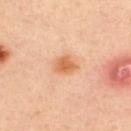Imaged during a routine full-body skin examination; the lesion was not biopsied and no histopathology is available.
A male subject aged 33–37.
Approximately 2.5 mm at its widest.
The lesion is on the upper back.
The total-body-photography lesion software estimated a lesion color around L≈62 a*≈24 b*≈39 in CIELAB, a lesion–skin lightness drop of about 10, and a normalized border contrast of about 7.5. And it measured a within-lesion color-variation index near 3/10.
A 15 mm close-up tile from a total-body photography series done for melanoma screening.
Captured under cross-polarized illumination.